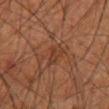The subject is a male in their 60s. The lesion is located on the mid back. A lesion tile, about 15 mm wide, cut from a 3D total-body photograph. Approximately 3 mm at its widest. Imaged with cross-polarized lighting.